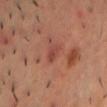Imaged during a routine full-body skin examination; the lesion was not biopsied and no histopathology is available.
A lesion tile, about 15 mm wide, cut from a 3D total-body photograph.
From the chest.
Approximately 2.5 mm at its widest.
A male patient, aged approximately 40.